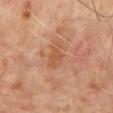Clinical impression: Captured during whole-body skin photography for melanoma surveillance; the lesion was not biopsied. Background: The lesion's longest dimension is about 3 mm. The patient is a male aged around 65. A lesion tile, about 15 mm wide, cut from a 3D total-body photograph. From the mid back.